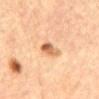Clinical summary:
The lesion is located on the chest. A female subject, aged 63–67. Longest diameter approximately 3 mm. A 15 mm crop from a total-body photograph taken for skin-cancer surveillance. Imaged with cross-polarized lighting.